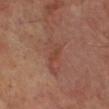The lesion was photographed on a routine skin check and not biopsied; there is no pathology result. About 2.5 mm across. Captured under cross-polarized illumination. An algorithmic analysis of the crop reported a footprint of about 2.5 mm² and an eccentricity of roughly 0.9. And it measured a border-irregularity rating of about 3.5/10, a within-lesion color-variation index near 0/10, and peripheral color asymmetry of about 0. The analysis additionally found a classifier nevus-likeness of about 0/100 and a detector confidence of about 100 out of 100 that the crop contains a lesion. A roughly 15 mm field-of-view crop from a total-body skin photograph. A male subject, aged approximately 70. Located on the leg.